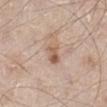Recorded during total-body skin imaging; not selected for excision or biopsy. A roughly 15 mm field-of-view crop from a total-body skin photograph. A male patient, approximately 60 years of age. This is a white-light tile. Approximately 3 mm at its widest. An algorithmic analysis of the crop reported a border-irregularity index near 2.5/10, a color-variation rating of about 6.5/10, and a peripheral color-asymmetry measure near 2.5. The software also gave an automated nevus-likeness rating near 30 out of 100. On the left lower leg.